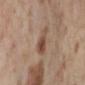Q: Was this lesion biopsied?
A: catalogued during a skin exam; not biopsied
Q: How was this image acquired?
A: ~15 mm tile from a whole-body skin photo
Q: What are the patient's age and sex?
A: female, roughly 55 years of age
Q: Lesion location?
A: the left lower leg